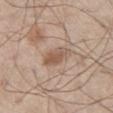follow-up: no biopsy performed (imaged during a skin exam) | lighting: white-light | automated metrics: an outline eccentricity of about 0.8 (0 = round, 1 = elongated) and a symmetry-axis asymmetry near 0.2; a mean CIELAB color near L≈55 a*≈17 b*≈28, about 10 CIELAB-L* units darker than the surrounding skin, and a normalized border contrast of about 7; internal color variation of about 3 on a 0–10 scale and radial color variation of about 1; a classifier nevus-likeness of about 45/100 and a detector confidence of about 100 out of 100 that the crop contains a lesion | site: the right thigh | subject: male, aged around 60 | acquisition: total-body-photography crop, ~15 mm field of view.